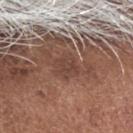Cropped from a total-body skin-imaging series; the visible field is about 15 mm.
A male subject, aged around 75.
Imaged with white-light lighting.
From the head or neck.
The lesion's longest dimension is about 3 mm.